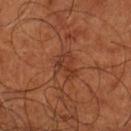No biopsy was performed on this lesion — it was imaged during a full skin examination and was not determined to be concerning. A 15 mm close-up extracted from a 3D total-body photography capture. The subject is aged 63 to 67. The lesion is located on the left lower leg.